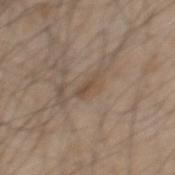Part of a total-body skin-imaging series; this lesion was reviewed on a skin check and was not flagged for biopsy.
On the mid back.
Captured under white-light illumination.
The recorded lesion diameter is about 2.5 mm.
A male patient, roughly 45 years of age.
A lesion tile, about 15 mm wide, cut from a 3D total-body photograph.